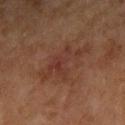The lesion was photographed on a routine skin check and not biopsied; there is no pathology result. This image is a 15 mm lesion crop taken from a total-body photograph. A female patient aged approximately 60. On the left forearm.